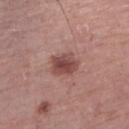Cropped from a whole-body photographic skin survey; the tile spans about 15 mm.
A female subject, aged around 75.
Located on the left lower leg.